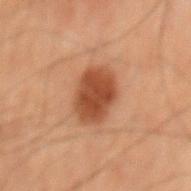{"image": {"source": "total-body photography crop", "field_of_view_mm": 15}, "site": "mid back", "automated_metrics": {"cielab_L": 36, "cielab_a": 21, "cielab_b": 28, "vs_skin_contrast_norm": 9.5}, "lighting": "cross-polarized", "patient": {"sex": "male", "age_approx": 70}}